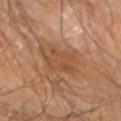Captured during whole-body skin photography for melanoma surveillance; the lesion was not biopsied. Located on the left forearm. A male subject, in their 60s. Approximately 4.5 mm at its widest. Captured under cross-polarized illumination. Automated tile analysis of the lesion measured an average lesion color of about L≈48 a*≈20 b*≈33 (CIELAB), about 6 CIELAB-L* units darker than the surrounding skin, and a lesion-to-skin contrast of about 5.5 (normalized; higher = more distinct). The analysis additionally found a border-irregularity index near 3/10, a color-variation rating of about 4/10, and a peripheral color-asymmetry measure near 1.5. The analysis additionally found an automated nevus-likeness rating near 0 out of 100 and a detector confidence of about 100 out of 100 that the crop contains a lesion. Cropped from a total-body skin-imaging series; the visible field is about 15 mm.